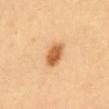Case summary:
* follow-up · no biopsy performed (imaged during a skin exam)
* subject · female, roughly 60 years of age
* lighting · cross-polarized
* TBP lesion metrics · an average lesion color of about L≈64 a*≈25 b*≈45 (CIELAB) and a lesion-to-skin contrast of about 9.5 (normalized; higher = more distinct)
* anatomic site · the abdomen
* diameter · about 3.5 mm
* image · ~15 mm tile from a whole-body skin photo